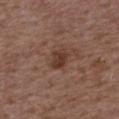The lesion was tiled from a total-body skin photograph and was not biopsied.
On the upper back.
A 15 mm close-up tile from a total-body photography series done for melanoma screening.
An algorithmic analysis of the crop reported a shape eccentricity near 0.8 and two-axis asymmetry of about 0.25. The analysis additionally found an average lesion color of about L≈37 a*≈20 b*≈26 (CIELAB), roughly 9 lightness units darker than nearby skin, and a normalized border contrast of about 8. It also reported a nevus-likeness score of about 55/100 and a lesion-detection confidence of about 100/100.
A male patient in their 50s.